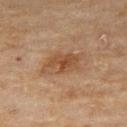Q: Was a biopsy performed?
A: catalogued during a skin exam; not biopsied
Q: How was this image acquired?
A: 15 mm crop, total-body photography
Q: What is the lesion's diameter?
A: about 5.5 mm
Q: Where on the body is the lesion?
A: the right thigh
Q: Who is the patient?
A: female, aged approximately 60